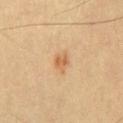The lesion was tiled from a total-body skin photograph and was not biopsied. A male subject, about 55 years old. A 15 mm close-up extracted from a 3D total-body photography capture. On the chest.